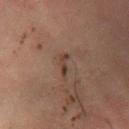Findings:
– follow-up: catalogued during a skin exam; not biopsied
– imaging modality: ~15 mm tile from a whole-body skin photo
– tile lighting: cross-polarized
– patient: female, aged approximately 80
– site: the left lower leg